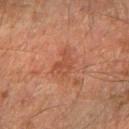follow-up — no biopsy performed (imaged during a skin exam); size — about 3.5 mm; acquisition — ~15 mm crop, total-body skin-cancer survey; tile lighting — cross-polarized illumination; anatomic site — the right forearm; subject — male, aged approximately 65.